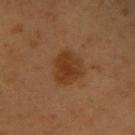workup = catalogued during a skin exam; not biopsied